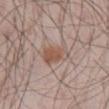This lesion was catalogued during total-body skin photography and was not selected for biopsy. A male subject roughly 55 years of age. This is a white-light tile. Located on the abdomen. The recorded lesion diameter is about 3.5 mm. Automated image analysis of the tile measured a lesion area of about 7.5 mm², a shape eccentricity near 0.7, and a shape-asymmetry score of about 0.3 (0 = symmetric). It also reported about 9 CIELAB-L* units darker than the surrounding skin and a normalized lesion–skin contrast near 7.5. The analysis additionally found border irregularity of about 3 on a 0–10 scale, internal color variation of about 2.5 on a 0–10 scale, and radial color variation of about 1. A close-up tile cropped from a whole-body skin photograph, about 15 mm across.